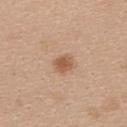The lesion's longest dimension is about 2.5 mm. Cropped from a total-body skin-imaging series; the visible field is about 15 mm. A female subject about 25 years old. The lesion is on the upper back.